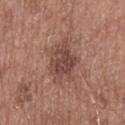Clinical impression:
The lesion was photographed on a routine skin check and not biopsied; there is no pathology result.
Clinical summary:
A region of skin cropped from a whole-body photographic capture, roughly 15 mm wide. The patient is a male roughly 50 years of age. From the mid back.